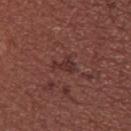Recorded during total-body skin imaging; not selected for excision or biopsy. The lesion is on the upper back. Captured under white-light illumination. A female subject approximately 25 years of age. The total-body-photography lesion software estimated a mean CIELAB color near L≈31 a*≈22 b*≈21, about 7 CIELAB-L* units darker than the surrounding skin, and a normalized lesion–skin contrast near 7. The lesion's longest dimension is about 2.5 mm. A 15 mm crop from a total-body photograph taken for skin-cancer surveillance.